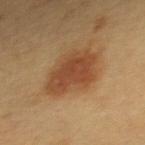This lesion was catalogued during total-body skin photography and was not selected for biopsy.
The patient is a female aged 28–32.
Imaged with cross-polarized lighting.
A 15 mm crop from a total-body photograph taken for skin-cancer surveillance.
About 5.5 mm across.
On the upper back.